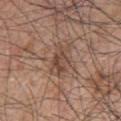Impression:
Imaged during a routine full-body skin examination; the lesion was not biopsied and no histopathology is available.
Background:
On the upper back. The patient is a male in their 70s. A roughly 15 mm field-of-view crop from a total-body skin photograph.